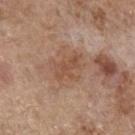Assessment: Recorded during total-body skin imaging; not selected for excision or biopsy. Context: A lesion tile, about 15 mm wide, cut from a 3D total-body photograph. The lesion is on the left forearm. Automated image analysis of the tile measured a footprint of about 5.5 mm², a shape eccentricity near 0.8, and a symmetry-axis asymmetry near 0.4. The analysis additionally found a color-variation rating of about 2/10 and peripheral color asymmetry of about 0.5. Approximately 3.5 mm at its widest. This is a white-light tile. A female patient, roughly 65 years of age.